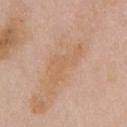workup: catalogued during a skin exam; not biopsied | patient: female, aged 68–72 | lighting: white-light | TBP lesion metrics: an outline eccentricity of about 0.95 (0 = round, 1 = elongated) and a symmetry-axis asymmetry near 0.55; a lesion color around L≈62 a*≈19 b*≈34 in CIELAB, roughly 5 lightness units darker than nearby skin, and a normalized lesion–skin contrast near 5 | size: ~6 mm (longest diameter) | site: the chest | image: total-body-photography crop, ~15 mm field of view.